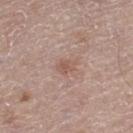Clinical impression:
Imaged during a routine full-body skin examination; the lesion was not biopsied and no histopathology is available.
Acquisition and patient details:
Automated image analysis of the tile measured an area of roughly 3 mm². The analysis additionally found a border-irregularity index near 3.5/10 and a color-variation rating of about 1.5/10. About 2.5 mm across. Located on the left thigh. Cropped from a total-body skin-imaging series; the visible field is about 15 mm. This is a white-light tile. The patient is a male aged around 80.